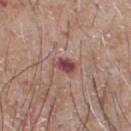| field | value |
|---|---|
| biopsy status | no biopsy performed (imaged during a skin exam) |
| automated metrics | a border-irregularity rating of about 2.5/10 and a peripheral color-asymmetry measure near 0.5; a classifier nevus-likeness of about 5/100 and a lesion-detection confidence of about 100/100 |
| lighting | white-light illumination |
| site | the front of the torso |
| lesion diameter | ≈3 mm |
| image | total-body-photography crop, ~15 mm field of view |
| subject | male, aged around 65 |